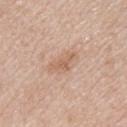biopsy status: catalogued during a skin exam; not biopsied
body site: the upper back
illumination: white-light illumination
lesion size: ~3 mm (longest diameter)
patient: female, approximately 45 years of age
imaging modality: ~15 mm tile from a whole-body skin photo